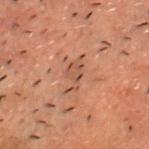body site: the front of the torso
patient: male, aged around 50
imaging modality: 15 mm crop, total-body photography